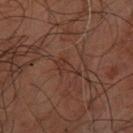Case summary:
- biopsy status — no biopsy performed (imaged during a skin exam)
- subject — male, in their mid-60s
- tile lighting — cross-polarized
- diameter — about 3 mm
- anatomic site — the left forearm
- automated lesion analysis — an average lesion color of about L≈30 a*≈19 b*≈23 (CIELAB), a lesion–skin lightness drop of about 5, and a normalized border contrast of about 5
- imaging modality — ~15 mm crop, total-body skin-cancer survey